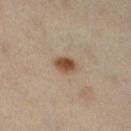The subject is a female aged approximately 40.
A region of skin cropped from a whole-body photographic capture, roughly 15 mm wide.
Imaged with cross-polarized lighting.
The lesion is located on the left leg.